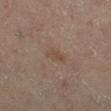Clinical impression:
Captured during whole-body skin photography for melanoma surveillance; the lesion was not biopsied.
Clinical summary:
Longest diameter approximately 2.5 mm. A male subject approximately 55 years of age. The lesion is on the right lower leg. A 15 mm close-up tile from a total-body photography series done for melanoma screening. Imaged with cross-polarized lighting.